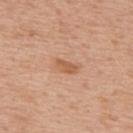notes = total-body-photography surveillance lesion; no biopsy
acquisition = total-body-photography crop, ~15 mm field of view
body site = the upper back
subject = male, aged around 70